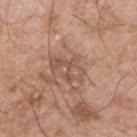follow-up: catalogued during a skin exam; not biopsied | imaging modality: 15 mm crop, total-body photography | automated metrics: an area of roughly 6.5 mm², an eccentricity of roughly 0.8, and a shape-asymmetry score of about 0.4 (0 = symmetric); a mean CIELAB color near L≈53 a*≈20 b*≈28, about 8 CIELAB-L* units darker than the surrounding skin, and a normalized lesion–skin contrast near 5.5; a nevus-likeness score of about 0/100 and a lesion-detection confidence of about 90/100 | subject: male, approximately 75 years of age | body site: the chest.